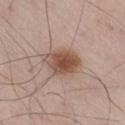Assessment: Part of a total-body skin-imaging series; this lesion was reviewed on a skin check and was not flagged for biopsy. Background: Longest diameter approximately 4.5 mm. A male subject, roughly 55 years of age. Located on the right thigh. The lesion-visualizer software estimated an average lesion color of about L≈51 a*≈19 b*≈27 (CIELAB) and a lesion–skin lightness drop of about 13. And it measured an automated nevus-likeness rating near 100 out of 100 and a lesion-detection confidence of about 100/100. A region of skin cropped from a whole-body photographic capture, roughly 15 mm wide. Imaged with white-light lighting.